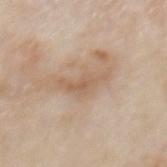follow-up: catalogued during a skin exam; not biopsied
TBP lesion metrics: a footprint of about 7.5 mm², a shape eccentricity near 0.9, and a symmetry-axis asymmetry near 0.5; an average lesion color of about L≈60 a*≈16 b*≈31 (CIELAB), about 8 CIELAB-L* units darker than the surrounding skin, and a lesion-to-skin contrast of about 6 (normalized; higher = more distinct); a border-irregularity index near 6.5/10 and a within-lesion color-variation index near 2.5/10
tile lighting: white-light illumination
body site: the mid back
subject: male, aged approximately 85 or older
diameter: about 5 mm
image source: total-body-photography crop, ~15 mm field of view